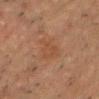  biopsy_status: not biopsied; imaged during a skin examination
  patient:
    sex: male
    age_approx: 45
  site: head or neck
  image:
    source: total-body photography crop
    field_of_view_mm: 15